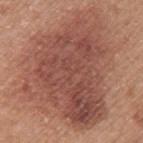The lesion was tiled from a total-body skin photograph and was not biopsied. Cropped from a whole-body photographic skin survey; the tile spans about 15 mm. The lesion is located on the left upper arm. A female subject about 50 years old. The tile uses white-light illumination. The recorded lesion diameter is about 13 mm.